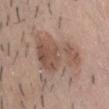follow-up: imaged on a skin check; not biopsied
lighting: white-light
size: about 6.5 mm
automated metrics: a lesion area of about 19 mm², an outline eccentricity of about 0.7 (0 = round, 1 = elongated), and a shape-asymmetry score of about 0.5 (0 = symmetric); a lesion–skin lightness drop of about 10 and a lesion-to-skin contrast of about 7 (normalized; higher = more distinct); a border-irregularity rating of about 6.5/10 and radial color variation of about 1.5
site: the front of the torso
patient: male, approximately 25 years of age
image source: total-body-photography crop, ~15 mm field of view